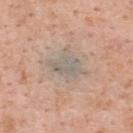Assessment: Recorded during total-body skin imaging; not selected for excision or biopsy.